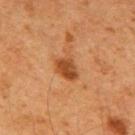Q: Was a biopsy performed?
A: total-body-photography surveillance lesion; no biopsy
Q: Patient demographics?
A: male, approximately 65 years of age
Q: Where on the body is the lesion?
A: the upper back
Q: What lighting was used for the tile?
A: cross-polarized
Q: Automated lesion metrics?
A: an area of roughly 6.5 mm², an eccentricity of roughly 0.65, and two-axis asymmetry of about 0.3; an average lesion color of about L≈40 a*≈22 b*≈34 (CIELAB), about 10 CIELAB-L* units darker than the surrounding skin, and a normalized border contrast of about 8.5
Q: How large is the lesion?
A: ~3.5 mm (longest diameter)
Q: How was this image acquired?
A: ~15 mm crop, total-body skin-cancer survey